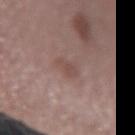notes = imaged on a skin check; not biopsied
anatomic site = the left forearm
lesion size = ≈2.5 mm
subject = female, roughly 50 years of age
illumination = white-light
acquisition = ~15 mm crop, total-body skin-cancer survey